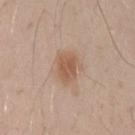Findings:
– workup: catalogued during a skin exam; not biopsied
– patient: male, aged 28–32
– body site: the left upper arm
– image source: total-body-photography crop, ~15 mm field of view
– lighting: white-light
– diameter: ~3.5 mm (longest diameter)